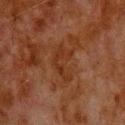follow-up: total-body-photography surveillance lesion; no biopsy
patient: male, approximately 80 years of age
image source: ~15 mm crop, total-body skin-cancer survey
diameter: about 4.5 mm
location: the upper back
TBP lesion metrics: an average lesion color of about L≈26 a*≈20 b*≈27 (CIELAB) and a normalized lesion–skin contrast near 6; an automated nevus-likeness rating near 0 out of 100